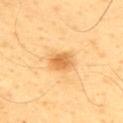The lesion was photographed on a routine skin check and not biopsied; there is no pathology result. This is a cross-polarized tile. About 3 mm across. A roughly 15 mm field-of-view crop from a total-body skin photograph. A male subject, in their mid- to late 50s. Automated image analysis of the tile measured a lesion area of about 6 mm², a shape eccentricity near 0.7, and two-axis asymmetry of about 0.1. It also reported an average lesion color of about L≈68 a*≈24 b*≈49 (CIELAB), roughly 12 lightness units darker than nearby skin, and a normalized border contrast of about 7.5. The software also gave a classifier nevus-likeness of about 90/100. Located on the upper back.